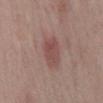workup = total-body-photography surveillance lesion; no biopsy
image source = ~15 mm crop, total-body skin-cancer survey
patient = male, approximately 70 years of age
lighting = white-light
lesion size = about 4 mm
site = the mid back